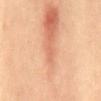Case summary:
- follow-up · no biopsy performed (imaged during a skin exam)
- subject · female, aged 33 to 37
- anatomic site · the mid back
- image · total-body-photography crop, ~15 mm field of view